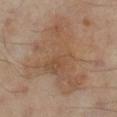notes = no biopsy performed (imaged during a skin exam)
image source = ~15 mm tile from a whole-body skin photo
site = the right lower leg
image-analysis metrics = an average lesion color of about L≈47 a*≈16 b*≈28 (CIELAB), roughly 7 lightness units darker than nearby skin, and a normalized lesion–skin contrast near 5.5; an automated nevus-likeness rating near 0 out of 100 and a detector confidence of about 100 out of 100 that the crop contains a lesion
diameter = ~9.5 mm (longest diameter)
patient = male, aged around 65
lighting = cross-polarized illumination